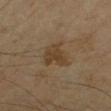Q: Was this lesion biopsied?
A: catalogued during a skin exam; not biopsied
Q: Where on the body is the lesion?
A: the right forearm
Q: What is the imaging modality?
A: total-body-photography crop, ~15 mm field of view
Q: Lesion size?
A: about 3.5 mm
Q: What are the patient's age and sex?
A: male, about 45 years old
Q: What did automated image analysis measure?
A: a lesion area of about 8 mm² and a shape eccentricity near 0.65; a mean CIELAB color near L≈39 a*≈14 b*≈30 and about 7 CIELAB-L* units darker than the surrounding skin; a nevus-likeness score of about 0/100 and a lesion-detection confidence of about 100/100